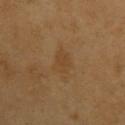Q: Was this lesion biopsied?
A: imaged on a skin check; not biopsied
Q: How was the tile lit?
A: cross-polarized illumination
Q: What are the patient's age and sex?
A: male, approximately 60 years of age
Q: What is the lesion's diameter?
A: ≈3 mm
Q: What is the imaging modality?
A: total-body-photography crop, ~15 mm field of view
Q: Where on the body is the lesion?
A: the upper back